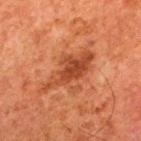Acquisition and patient details:
The lesion is on the upper back. The subject is a male aged 78 to 82. This is a cross-polarized tile. This image is a 15 mm lesion crop taken from a total-body photograph. Automated image analysis of the tile measured a lesion area of about 13 mm², a shape eccentricity near 0.9, and two-axis asymmetry of about 0.35. And it measured a mean CIELAB color near L≈37 a*≈25 b*≈32, about 9 CIELAB-L* units darker than the surrounding skin, and a normalized lesion–skin contrast near 7.5. The analysis additionally found border irregularity of about 5 on a 0–10 scale, a within-lesion color-variation index near 4/10, and radial color variation of about 1.5.